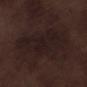Clinical impression: No biopsy was performed on this lesion — it was imaged during a full skin examination and was not determined to be concerning. Background: A male patient, aged 68–72. On the leg. A 15 mm close-up tile from a total-body photography series done for melanoma screening. Longest diameter approximately 10 mm. The lesion-visualizer software estimated an eccentricity of roughly 0.9 and a symmetry-axis asymmetry near 0.25. The analysis additionally found a lesion color around L≈17 a*≈13 b*≈13 in CIELAB, about 4 CIELAB-L* units darker than the surrounding skin, and a lesion-to-skin contrast of about 7 (normalized; higher = more distinct). Imaged with white-light lighting.